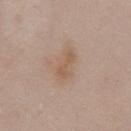biopsy status: catalogued during a skin exam; not biopsied | anatomic site: the chest | subject: male, in their mid- to late 50s | illumination: white-light | imaging modality: ~15 mm tile from a whole-body skin photo | size: about 4 mm.